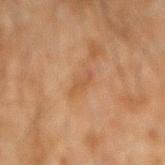| key | value |
|---|---|
| follow-up | imaged on a skin check; not biopsied |